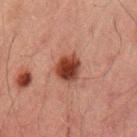workup: total-body-photography surveillance lesion; no biopsy
subject: male, aged 48 to 52
acquisition: ~15 mm crop, total-body skin-cancer survey
location: the left upper arm
image-analysis metrics: an area of roughly 8.5 mm², an outline eccentricity of about 0.6 (0 = round, 1 = elongated), and a shape-asymmetry score of about 0.2 (0 = symmetric); about 13 CIELAB-L* units darker than the surrounding skin; a border-irregularity rating of about 2/10, a within-lesion color-variation index near 5/10, and radial color variation of about 1.5; an automated nevus-likeness rating near 100 out of 100 and a lesion-detection confidence of about 100/100
tile lighting: cross-polarized illumination
size: about 3.5 mm